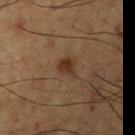image source = ~15 mm tile from a whole-body skin photo
location = the left upper arm
subject = male, aged 58 to 62
lighting = cross-polarized
lesion diameter = about 2.5 mm
TBP lesion metrics = an area of roughly 4 mm² and a shape eccentricity near 0.6; a border-irregularity index near 3/10, internal color variation of about 2 on a 0–10 scale, and a peripheral color-asymmetry measure near 0.5; an automated nevus-likeness rating near 80 out of 100 and a lesion-detection confidence of about 100/100